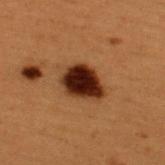biopsy status: imaged on a skin check; not biopsied | lighting: cross-polarized | TBP lesion metrics: a color-variation rating of about 5.5/10 and a peripheral color-asymmetry measure near 1 | size: about 4.5 mm | body site: the upper back | image: ~15 mm tile from a whole-body skin photo | subject: male, approximately 50 years of age.